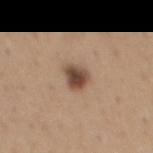This lesion was catalogued during total-body skin photography and was not selected for biopsy. An algorithmic analysis of the crop reported a border-irregularity rating of about 2.5/10, internal color variation of about 4.5 on a 0–10 scale, and radial color variation of about 1. The analysis additionally found a nevus-likeness score of about 95/100 and a lesion-detection confidence of about 100/100. This image is a 15 mm lesion crop taken from a total-body photograph. From the chest. A male subject roughly 65 years of age.